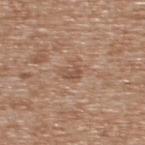Captured during whole-body skin photography for melanoma surveillance; the lesion was not biopsied. From the upper back. A male patient about 65 years old. Approximately 2.5 mm at its widest. A region of skin cropped from a whole-body photographic capture, roughly 15 mm wide.